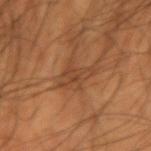<tbp_lesion>
<biopsy_status>not biopsied; imaged during a skin examination</biopsy_status>
<automated_metrics>
  <eccentricity>0.9</eccentricity>
  <shape_asymmetry>0.5</shape_asymmetry>
  <nevus_likeness_0_100>0</nevus_likeness_0_100>
  <lesion_detection_confidence_0_100>55</lesion_detection_confidence_0_100>
</automated_metrics>
<image>
  <source>total-body photography crop</source>
  <field_of_view_mm>15</field_of_view_mm>
</image>
<lesion_size>
  <long_diameter_mm_approx>4.0</long_diameter_mm_approx>
</lesion_size>
<patient>
  <sex>male</sex>
  <age_approx>70</age_approx>
</patient>
<lighting>cross-polarized</lighting>
<site>right upper arm</site>
</tbp_lesion>